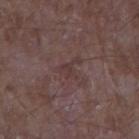follow-up = catalogued during a skin exam; not biopsied
size = ≈3 mm
patient = male, aged around 65
acquisition = 15 mm crop, total-body photography
location = the left forearm
tile lighting = white-light
TBP lesion metrics = an automated nevus-likeness rating near 0 out of 100 and lesion-presence confidence of about 85/100